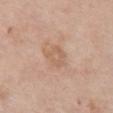Q: Was this lesion biopsied?
A: no biopsy performed (imaged during a skin exam)
Q: How large is the lesion?
A: ≈3 mm
Q: How was this image acquired?
A: ~15 mm tile from a whole-body skin photo
Q: Who is the patient?
A: female, approximately 65 years of age
Q: Lesion location?
A: the front of the torso